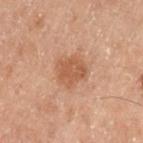Impression:
The lesion was tiled from a total-body skin photograph and was not biopsied.